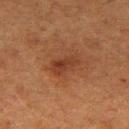Q: Was this lesion biopsied?
A: catalogued during a skin exam; not biopsied
Q: What lighting was used for the tile?
A: cross-polarized illumination
Q: What is the imaging modality?
A: 15 mm crop, total-body photography
Q: What is the anatomic site?
A: the right thigh
Q: Patient demographics?
A: female, in their 50s
Q: What did automated image analysis measure?
A: two-axis asymmetry of about 0.25; a within-lesion color-variation index near 3/10 and peripheral color asymmetry of about 1; a classifier nevus-likeness of about 20/100 and a lesion-detection confidence of about 100/100